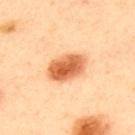Recorded during total-body skin imaging; not selected for excision or biopsy.
This image is a 15 mm lesion crop taken from a total-body photograph.
Captured under cross-polarized illumination.
The lesion is on the upper back.
The subject is a male aged 43 to 47.